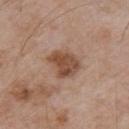{"biopsy_status": "not biopsied; imaged during a skin examination", "automated_metrics": {"cielab_L": 48, "cielab_a": 20, "cielab_b": 29, "vs_skin_darker_L": 12.0, "vs_skin_contrast_norm": 9.0, "nevus_likeness_0_100": 30, "lesion_detection_confidence_0_100": 100}, "site": "upper back", "patient": {"sex": "male", "age_approx": 55}, "image": {"source": "total-body photography crop", "field_of_view_mm": 15}, "lesion_size": {"long_diameter_mm_approx": 3.5}}